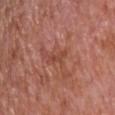Captured during whole-body skin photography for melanoma surveillance; the lesion was not biopsied.
A region of skin cropped from a whole-body photographic capture, roughly 15 mm wide.
A male patient approximately 65 years of age.
The lesion is on the front of the torso.